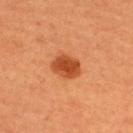  biopsy_status: not biopsied; imaged during a skin examination
  lighting: cross-polarized
  lesion_size:
    long_diameter_mm_approx: 3.5
  image:
    source: total-body photography crop
    field_of_view_mm: 15
  patient:
    sex: female
    age_approx: 50